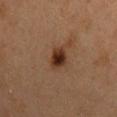<lesion>
  <biopsy_status>not biopsied; imaged during a skin examination</biopsy_status>
  <patient>
    <sex>female</sex>
    <age_approx>40</age_approx>
  </patient>
  <site>mid back</site>
  <image>
    <source>total-body photography crop</source>
    <field_of_view_mm>15</field_of_view_mm>
  </image>
</lesion>